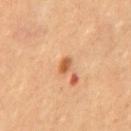Findings:
– workup — no biopsy performed (imaged during a skin exam)
– diameter — ≈2 mm
– automated lesion analysis — a footprint of about 2.5 mm² and two-axis asymmetry of about 0.15
– body site — the chest
– image source — 15 mm crop, total-body photography
– patient — female, aged 53 to 57